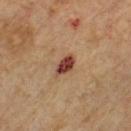A male subject aged around 65. Measured at roughly 3 mm in maximum diameter. From the chest. A 15 mm close-up tile from a total-body photography series done for melanoma screening. The tile uses cross-polarized illumination.